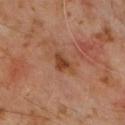Assessment: The lesion was tiled from a total-body skin photograph and was not biopsied. Context: The total-body-photography lesion software estimated a footprint of about 4.5 mm² and a shape eccentricity near 0.7. The software also gave a border-irregularity index near 4/10, a color-variation rating of about 2.5/10, and radial color variation of about 1. It also reported a classifier nevus-likeness of about 15/100 and lesion-presence confidence of about 100/100. A region of skin cropped from a whole-body photographic capture, roughly 15 mm wide. The patient is a male about 60 years old. This is a cross-polarized tile. The lesion is located on the chest.